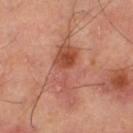Case summary:
* follow-up: imaged on a skin check; not biopsied
* lesion diameter: about 8 mm
* site: the right thigh
* imaging modality: ~15 mm crop, total-body skin-cancer survey